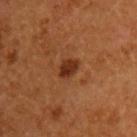Recorded during total-body skin imaging; not selected for excision or biopsy. Located on the front of the torso. A close-up tile cropped from a whole-body skin photograph, about 15 mm across. The patient is a male aged 53–57. Automated image analysis of the tile measured a footprint of about 4 mm² and a symmetry-axis asymmetry near 0.2. The software also gave internal color variation of about 3 on a 0–10 scale and radial color variation of about 1. The recorded lesion diameter is about 2.5 mm.